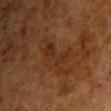– illumination — cross-polarized
– patient — male, aged approximately 60
– image — total-body-photography crop, ~15 mm field of view
– site — the chest